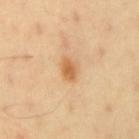Q: Was this lesion biopsied?
A: imaged on a skin check; not biopsied